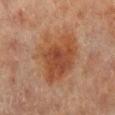Captured during whole-body skin photography for melanoma surveillance; the lesion was not biopsied.
A 15 mm crop from a total-body photograph taken for skin-cancer surveillance.
Measured at roughly 6.5 mm in maximum diameter.
From the right lower leg.
A female patient approximately 80 years of age.
The tile uses cross-polarized illumination.
Automated image analysis of the tile measured an average lesion color of about L≈41 a*≈21 b*≈30 (CIELAB) and a normalized border contrast of about 7.5.